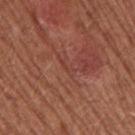Notes:
- follow-up — catalogued during a skin exam; not biopsied
- automated lesion analysis — an outline eccentricity of about 0.95 (0 = round, 1 = elongated) and a symmetry-axis asymmetry near 0.45; an average lesion color of about L≈42 a*≈26 b*≈28 (CIELAB), a lesion–skin lightness drop of about 5, and a normalized lesion–skin contrast near 4; a classifier nevus-likeness of about 0/100 and a lesion-detection confidence of about 5/100
- location — the right upper arm
- subject — male, aged approximately 55
- illumination — white-light
- acquisition — total-body-photography crop, ~15 mm field of view
- size — ~2.5 mm (longest diameter)